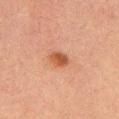notes=total-body-photography surveillance lesion; no biopsy | image=~15 mm crop, total-body skin-cancer survey | site=the arm | subject=male, about 50 years old.